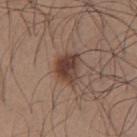Case summary:
- notes — catalogued during a skin exam; not biopsied
- TBP lesion metrics — an area of roughly 8 mm² and a symmetry-axis asymmetry near 0.25; a mean CIELAB color near L≈40 a*≈18 b*≈24 and roughly 12 lightness units darker than nearby skin; a detector confidence of about 100 out of 100 that the crop contains a lesion
- lighting — white-light
- patient — male, about 25 years old
- site — the chest
- diameter — ~3.5 mm (longest diameter)
- imaging modality — ~15 mm tile from a whole-body skin photo